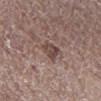Imaged during a routine full-body skin examination; the lesion was not biopsied and no histopathology is available. The recorded lesion diameter is about 3 mm. The subject is a male roughly 70 years of age. This is a white-light tile. From the right lower leg. A region of skin cropped from a whole-body photographic capture, roughly 15 mm wide.